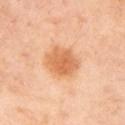Imaged during a routine full-body skin examination; the lesion was not biopsied and no histopathology is available.
A roughly 15 mm field-of-view crop from a total-body skin photograph.
The recorded lesion diameter is about 4 mm.
Captured under cross-polarized illumination.
The lesion-visualizer software estimated a lesion–skin lightness drop of about 11. The software also gave a nevus-likeness score of about 90/100 and a lesion-detection confidence of about 100/100.
The lesion is located on the left upper arm.
The patient is a female about 45 years old.